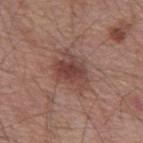This lesion was catalogued during total-body skin photography and was not selected for biopsy.
This is a white-light tile.
The subject is a male about 55 years old.
Automated image analysis of the tile measured a lesion area of about 12 mm², a shape eccentricity near 0.7, and a symmetry-axis asymmetry near 0.35. The analysis additionally found a mean CIELAB color near L≈44 a*≈21 b*≈23, roughly 9 lightness units darker than nearby skin, and a lesion-to-skin contrast of about 7.5 (normalized; higher = more distinct). And it measured a border-irregularity rating of about 4/10 and peripheral color asymmetry of about 1.5.
About 5 mm across.
A lesion tile, about 15 mm wide, cut from a 3D total-body photograph.
From the mid back.